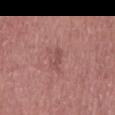Impression:
No biopsy was performed on this lesion — it was imaged during a full skin examination and was not determined to be concerning.
Context:
This image is a 15 mm lesion crop taken from a total-body photograph. Located on the mid back. A male patient in their 60s.